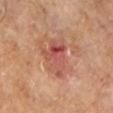• notes · catalogued during a skin exam; not biopsied
• size · ≈5.5 mm
• lighting · cross-polarized
• image · total-body-photography crop, ~15 mm field of view
• patient · male, aged around 65
• TBP lesion metrics · a symmetry-axis asymmetry near 0.35; a mean CIELAB color near L≈51 a*≈27 b*≈28, a lesion–skin lightness drop of about 9, and a normalized lesion–skin contrast near 7; a within-lesion color-variation index near 10/10 and a peripheral color-asymmetry measure near 3.5